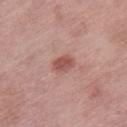follow-up — total-body-photography surveillance lesion; no biopsy
diameter — about 2.5 mm
imaging modality — 15 mm crop, total-body photography
subject — female, in their mid- to late 60s
automated lesion analysis — a lesion area of about 4 mm², a shape eccentricity near 0.7, and a shape-asymmetry score of about 0.25 (0 = symmetric); a border-irregularity rating of about 2/10, a within-lesion color-variation index near 1.5/10, and radial color variation of about 0.5
location — the right thigh